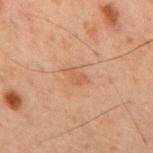subject — male, about 60 years old | TBP lesion metrics — a footprint of about 3.5 mm², a shape eccentricity near 0.8, and two-axis asymmetry of about 0.35; a peripheral color-asymmetry measure near 0.5; a detector confidence of about 100 out of 100 that the crop contains a lesion | lighting — cross-polarized | location — the back | acquisition — 15 mm crop, total-body photography | lesion diameter — ≈2.5 mm.